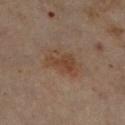No biopsy was performed on this lesion — it was imaged during a full skin examination and was not determined to be concerning. Automated tile analysis of the lesion measured a lesion area of about 10 mm², a shape eccentricity near 0.8, and a symmetry-axis asymmetry near 0.25. The analysis additionally found an average lesion color of about L≈42 a*≈17 b*≈28 (CIELAB), a lesion–skin lightness drop of about 7, and a normalized lesion–skin contrast near 7. And it measured border irregularity of about 3 on a 0–10 scale, a within-lesion color-variation index near 4.5/10, and radial color variation of about 1.5. The software also gave lesion-presence confidence of about 100/100. A close-up tile cropped from a whole-body skin photograph, about 15 mm across. The tile uses cross-polarized illumination. The subject is a female aged 58 to 62. Located on the right lower leg. The lesion's longest dimension is about 4.5 mm.